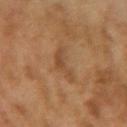Assessment: This lesion was catalogued during total-body skin photography and was not selected for biopsy. Context: On the left upper arm. The subject is a female aged around 60. The lesion's longest dimension is about 3.5 mm. A 15 mm close-up tile from a total-body photography series done for melanoma screening. This is a cross-polarized tile.